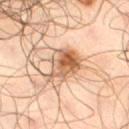Clinical impression:
Recorded during total-body skin imaging; not selected for excision or biopsy.
Acquisition and patient details:
A 15 mm close-up tile from a total-body photography series done for melanoma screening. From the right thigh. The tile uses cross-polarized illumination. The patient is a male in their mid-60s. Longest diameter approximately 5 mm.